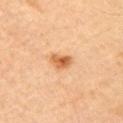The lesion was tiled from a total-body skin photograph and was not biopsied.
Located on the right upper arm.
An algorithmic analysis of the crop reported a border-irregularity index near 2.5/10, a color-variation rating of about 4.5/10, and peripheral color asymmetry of about 1.5.
A lesion tile, about 15 mm wide, cut from a 3D total-body photograph.
This is a cross-polarized tile.
A male patient, in their mid-40s.